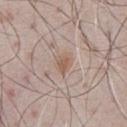Findings:
- biopsy status: catalogued during a skin exam; not biopsied
- automated metrics: a footprint of about 4 mm², an eccentricity of roughly 0.7, and two-axis asymmetry of about 0.2; an average lesion color of about L≈58 a*≈16 b*≈26 (CIELAB), about 8 CIELAB-L* units darker than the surrounding skin, and a normalized lesion–skin contrast near 6.5; internal color variation of about 2 on a 0–10 scale and radial color variation of about 0.5; an automated nevus-likeness rating near 45 out of 100
- patient: male, aged 68 to 72
- site: the front of the torso
- image source: ~15 mm tile from a whole-body skin photo
- lighting: white-light illumination